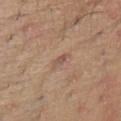| field | value |
|---|---|
| biopsy status | no biopsy performed (imaged during a skin exam) |
| anatomic site | the abdomen |
| illumination | white-light |
| image-analysis metrics | an eccentricity of roughly 0.9 and two-axis asymmetry of about 0.4; an average lesion color of about L≈53 a*≈18 b*≈27 (CIELAB), roughly 7 lightness units darker than nearby skin, and a normalized lesion–skin contrast near 5.5; a classifier nevus-likeness of about 0/100 and a lesion-detection confidence of about 100/100 |
| image | total-body-photography crop, ~15 mm field of view |
| patient | female, aged around 25 |
| lesion diameter | about 2.5 mm |